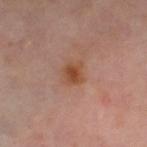{"biopsy_status": "not biopsied; imaged during a skin examination", "image": {"source": "total-body photography crop", "field_of_view_mm": 15}, "site": "left thigh", "lighting": "cross-polarized", "patient": {"sex": "female", "age_approx": 50}, "automated_metrics": {"vs_skin_darker_L": 8.0, "vs_skin_contrast_norm": 8.0, "border_irregularity_0_10": 2.5, "color_variation_0_10": 3.5, "peripheral_color_asymmetry": 1.0, "nevus_likeness_0_100": 55, "lesion_detection_confidence_0_100": 100}}